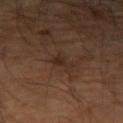No biopsy was performed on this lesion — it was imaged during a full skin examination and was not determined to be concerning. A male patient, aged approximately 55. Cropped from a whole-body photographic skin survey; the tile spans about 15 mm. From the left forearm.